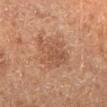No biopsy was performed on this lesion — it was imaged during a full skin examination and was not determined to be concerning.
A roughly 15 mm field-of-view crop from a total-body skin photograph.
The lesion-visualizer software estimated a footprint of about 12 mm², an outline eccentricity of about 0.65 (0 = round, 1 = elongated), and a symmetry-axis asymmetry near 0.3. It also reported an average lesion color of about L≈52 a*≈22 b*≈32 (CIELAB) and a normalized lesion–skin contrast near 5.5. It also reported a border-irregularity index near 4.5/10, a color-variation rating of about 3/10, and a peripheral color-asymmetry measure near 1.
This is a cross-polarized tile.
A male subject in their 60s.
Measured at roughly 4.5 mm in maximum diameter.
The lesion is located on the right lower leg.